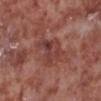Q: Was this lesion biopsied?
A: catalogued during a skin exam; not biopsied
Q: Lesion location?
A: the leg
Q: How was this image acquired?
A: 15 mm crop, total-body photography
Q: How was the tile lit?
A: white-light illumination
Q: Patient demographics?
A: male, aged around 55